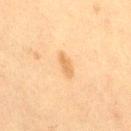Recorded during total-body skin imaging; not selected for excision or biopsy. A 15 mm crop from a total-body photograph taken for skin-cancer surveillance. Located on the right thigh. A female patient, aged around 40.